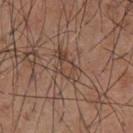biopsy_status: not biopsied; imaged during a skin examination
patient:
  sex: male
  age_approx: 55
lesion_size:
  long_diameter_mm_approx: 4.5
site: front of the torso
image:
  source: total-body photography crop
  field_of_view_mm: 15
automated_metrics:
  cielab_L: 44
  cielab_a: 17
  cielab_b: 26
  vs_skin_darker_L: 7.0
  vs_skin_contrast_norm: 5.5
  border_irregularity_0_10: 4.5
  color_variation_0_10: 5.5
  peripheral_color_asymmetry: 2.0
  nevus_likeness_0_100: 0